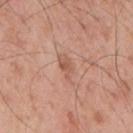Q: Was this lesion biopsied?
A: catalogued during a skin exam; not biopsied
Q: What is the anatomic site?
A: the mid back
Q: What is the imaging modality?
A: ~15 mm tile from a whole-body skin photo
Q: Patient demographics?
A: male, aged approximately 55